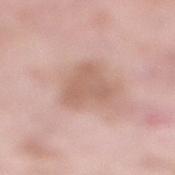Assessment:
Recorded during total-body skin imaging; not selected for excision or biopsy.
Background:
Automated image analysis of the tile measured a lesion–skin lightness drop of about 9. The analysis additionally found a border-irregularity index near 4/10, a within-lesion color-variation index near 2/10, and peripheral color asymmetry of about 0.5. A roughly 15 mm field-of-view crop from a total-body skin photograph. The lesion is on the right lower leg. A female patient aged 68–72. This is a white-light tile.